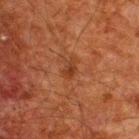Impression: The lesion was photographed on a routine skin check and not biopsied; there is no pathology result. Acquisition and patient details: A close-up tile cropped from a whole-body skin photograph, about 15 mm across. Captured under cross-polarized illumination. On the upper back. About 2.5 mm across. The subject is a male roughly 60 years of age.